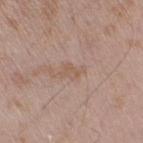* follow-up: catalogued during a skin exam; not biopsied
* subject: male, aged around 50
* acquisition: ~15 mm tile from a whole-body skin photo
* lesion size: ~2.5 mm (longest diameter)
* location: the right upper arm